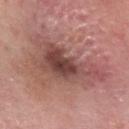Image and clinical context:
The lesion is located on the head or neck. The total-body-photography lesion software estimated a footprint of about 30 mm², a shape eccentricity near 0.85, and a shape-asymmetry score of about 0.45 (0 = symmetric). And it measured an automated nevus-likeness rating near 0 out of 100 and a detector confidence of about 100 out of 100 that the crop contains a lesion. A male subject, roughly 80 years of age. This image is a 15 mm lesion crop taken from a total-body photograph.
Diagnosis:
Histopathology of the biopsied lesion showed a melanoma in situ, classified as a malignancy.